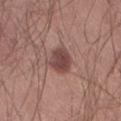No biopsy was performed on this lesion — it was imaged during a full skin examination and was not determined to be concerning. A male subject aged 23 to 27. Measured at roughly 3.5 mm in maximum diameter. The lesion is on the lower back. This image is a 15 mm lesion crop taken from a total-body photograph. This is a white-light tile.